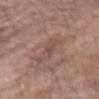<lesion>
<biopsy_status>not biopsied; imaged during a skin examination</biopsy_status>
<image>
  <source>total-body photography crop</source>
  <field_of_view_mm>15</field_of_view_mm>
</image>
<lesion_size>
  <long_diameter_mm_approx>4.5</long_diameter_mm_approx>
</lesion_size>
<lighting>white-light</lighting>
<site>right forearm</site>
<patient>
  <sex>male</sex>
  <age_approx>50</age_approx>
</patient>
</lesion>